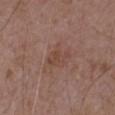Q: Is there a histopathology result?
A: catalogued during a skin exam; not biopsied
Q: How was this image acquired?
A: 15 mm crop, total-body photography
Q: Lesion location?
A: the right upper arm
Q: How was the tile lit?
A: white-light
Q: Who is the patient?
A: male, aged approximately 50